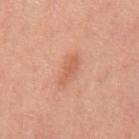biopsy status = no biopsy performed (imaged during a skin exam); patient = male, roughly 50 years of age; image = total-body-photography crop, ~15 mm field of view; lesion diameter = about 3.5 mm; lighting = white-light; site = the mid back.